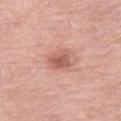| key | value |
|---|---|
| biopsy status | no biopsy performed (imaged during a skin exam) |
| image source | 15 mm crop, total-body photography |
| automated metrics | an outline eccentricity of about 0.55 (0 = round, 1 = elongated) and a shape-asymmetry score of about 0.25 (0 = symmetric) |
| size | ≈2.5 mm |
| location | the left thigh |
| subject | female, in their 70s |
| illumination | white-light illumination |